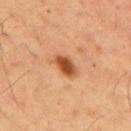Q: Is there a histopathology result?
A: catalogued during a skin exam; not biopsied
Q: What kind of image is this?
A: ~15 mm crop, total-body skin-cancer survey
Q: What is the anatomic site?
A: the right upper arm
Q: How large is the lesion?
A: ≈3 mm
Q: Illumination type?
A: cross-polarized illumination
Q: Who is the patient?
A: male, aged around 55